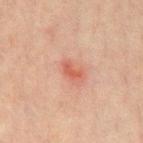biopsy status: catalogued during a skin exam; not biopsied | patient: male, about 70 years old | imaging modality: ~15 mm tile from a whole-body skin photo | lesion size: ≈2.5 mm | illumination: cross-polarized | site: the mid back.